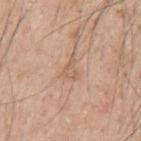location = the arm | patient = male, aged around 60 | image = total-body-photography crop, ~15 mm field of view | diameter = ~2.5 mm (longest diameter).